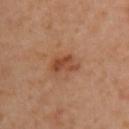The lesion was tiled from a total-body skin photograph and was not biopsied.
An algorithmic analysis of the crop reported a lesion area of about 5 mm², an outline eccentricity of about 0.8 (0 = round, 1 = elongated), and a symmetry-axis asymmetry near 0.35. And it measured a nevus-likeness score of about 45/100 and a lesion-detection confidence of about 100/100.
Cropped from a total-body skin-imaging series; the visible field is about 15 mm.
A female patient in their 60s.
Approximately 3.5 mm at its widest.
This is a cross-polarized tile.
From the right upper arm.